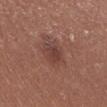The lesion was tiled from a total-body skin photograph and was not biopsied.
The lesion's longest dimension is about 3.5 mm.
An algorithmic analysis of the crop reported border irregularity of about 1.5 on a 0–10 scale, internal color variation of about 5 on a 0–10 scale, and peripheral color asymmetry of about 1.5.
A 15 mm crop from a total-body photograph taken for skin-cancer surveillance.
The patient is a female in their mid- to late 20s.
The lesion is located on the lower back.
Imaged with white-light lighting.